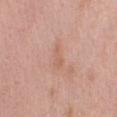  lighting: white-light
  lesion_size:
    long_diameter_mm_approx: 3.0
  automated_metrics:
    cielab_L: 61
    cielab_a: 22
    cielab_b: 30
    vs_skin_darker_L: 6.0
    vs_skin_contrast_norm: 5.0
    color_variation_0_10: 0.5
    peripheral_color_asymmetry: 0.0
    nevus_likeness_0_100: 0
    lesion_detection_confidence_0_100: 100
  image:
    source: total-body photography crop
    field_of_view_mm: 15
  site: arm
  patient:
    sex: male
    age_approx: 55